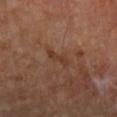The lesion was photographed on a routine skin check and not biopsied; there is no pathology result. A female subject aged 63–67. A 15 mm crop from a total-body photograph taken for skin-cancer surveillance. The lesion is located on the right forearm. Automated image analysis of the tile measured a lesion area of about 3 mm² and an outline eccentricity of about 0.95 (0 = round, 1 = elongated). And it measured a mean CIELAB color near L≈38 a*≈21 b*≈29, roughly 6 lightness units darker than nearby skin, and a lesion-to-skin contrast of about 6 (normalized; higher = more distinct). The analysis additionally found a border-irregularity index near 4.5/10 and a within-lesion color-variation index near 0/10. The analysis additionally found a classifier nevus-likeness of about 0/100 and a lesion-detection confidence of about 100/100. This is a cross-polarized tile. Measured at roughly 3.5 mm in maximum diameter.